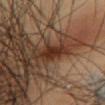This lesion was catalogued during total-body skin photography and was not selected for biopsy. A 15 mm crop from a total-body photograph taken for skin-cancer surveillance. Automated image analysis of the tile measured a mean CIELAB color near L≈24 a*≈17 b*≈23, about 9 CIELAB-L* units darker than the surrounding skin, and a lesion-to-skin contrast of about 9.5 (normalized; higher = more distinct). And it measured border irregularity of about 2 on a 0–10 scale. The software also gave a lesion-detection confidence of about 100/100. On the abdomen. The tile uses cross-polarized illumination. A male subject, roughly 55 years of age.